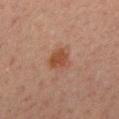location: the back; lesion diameter: ≈3 mm; image: total-body-photography crop, ~15 mm field of view; patient: male, approximately 30 years of age; tile lighting: cross-polarized illumination.